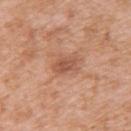From the upper back.
A female subject, aged approximately 40.
Imaged with white-light lighting.
About 3 mm across.
The total-body-photography lesion software estimated an area of roughly 5 mm². And it measured a mean CIELAB color near L≈54 a*≈24 b*≈32 and roughly 9 lightness units darker than nearby skin. It also reported a within-lesion color-variation index near 2/10.
A 15 mm close-up tile from a total-body photography series done for melanoma screening.